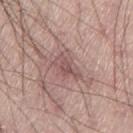Captured during whole-body skin photography for melanoma surveillance; the lesion was not biopsied.
Cropped from a whole-body photographic skin survey; the tile spans about 15 mm.
Located on the left thigh.
The patient is a male approximately 45 years of age.